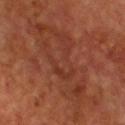body site: the chest | subject: male, approximately 65 years of age | illumination: cross-polarized illumination | size: ≈13 mm | TBP lesion metrics: an outline eccentricity of about 0.95 (0 = round, 1 = elongated) and a symmetry-axis asymmetry near 0.45; a nevus-likeness score of about 0/100 and a detector confidence of about 60 out of 100 that the crop contains a lesion | image: total-body-photography crop, ~15 mm field of view.